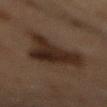workup: catalogued during a skin exam; not biopsied | anatomic site: the mid back | subject: male, aged around 85 or older | imaging modality: 15 mm crop, total-body photography.